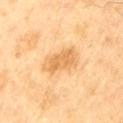notes — imaged on a skin check; not biopsied
lighting — cross-polarized illumination
diameter — about 5 mm
image source — 15 mm crop, total-body photography
anatomic site — the left thigh
subject — male, in their 70s